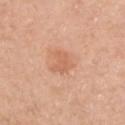notes — no biopsy performed (imaged during a skin exam)
site — the chest
image source — 15 mm crop, total-body photography
patient — male, in their 40s
TBP lesion metrics — a footprint of about 5 mm², a shape eccentricity near 0.7, and two-axis asymmetry of about 0.4; a nevus-likeness score of about 10/100 and lesion-presence confidence of about 100/100
illumination — white-light
diameter — ≈3.5 mm